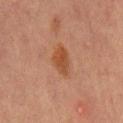| feature | finding |
|---|---|
| workup | imaged on a skin check; not biopsied |
| automated lesion analysis | a within-lesion color-variation index near 2.5/10; a classifier nevus-likeness of about 80/100 and lesion-presence confidence of about 100/100 |
| tile lighting | cross-polarized illumination |
| subject | male, aged 63 to 67 |
| image | total-body-photography crop, ~15 mm field of view |
| body site | the front of the torso |